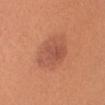No biopsy was performed on this lesion — it was imaged during a full skin examination and was not determined to be concerning.
The patient is a female about 25 years old.
The lesion-visualizer software estimated a mean CIELAB color near L≈54 a*≈27 b*≈33, a lesion–skin lightness drop of about 8, and a normalized lesion–skin contrast near 6.
On the head or neck.
Cropped from a whole-body photographic skin survey; the tile spans about 15 mm.
This is a white-light tile.
The recorded lesion diameter is about 5 mm.